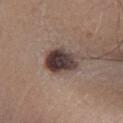Recorded during total-body skin imaging; not selected for excision or biopsy. The lesion is on the left lower leg. This is a white-light tile. A male subject, aged 63–67. A 15 mm close-up tile from a total-body photography series done for melanoma screening. Automated tile analysis of the lesion measured an average lesion color of about L≈38 a*≈15 b*≈18 (CIELAB), a lesion–skin lightness drop of about 17, and a normalized border contrast of about 14. The analysis additionally found border irregularity of about 2 on a 0–10 scale, internal color variation of about 7.5 on a 0–10 scale, and a peripheral color-asymmetry measure near 2.5.